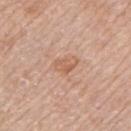Q: Was a biopsy performed?
A: catalogued during a skin exam; not biopsied
Q: Automated lesion metrics?
A: a footprint of about 4.5 mm² and a symmetry-axis asymmetry near 0.3; a nevus-likeness score of about 0/100 and a detector confidence of about 100 out of 100 that the crop contains a lesion
Q: What is the lesion's diameter?
A: ≈3 mm
Q: Patient demographics?
A: male, aged approximately 65
Q: What is the imaging modality?
A: ~15 mm crop, total-body skin-cancer survey
Q: Lesion location?
A: the left upper arm